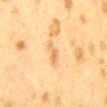Findings:
- workup: imaged on a skin check; not biopsied
- lighting: cross-polarized illumination
- site: the mid back
- patient: female, aged 38–42
- automated lesion analysis: a lesion color around L≈60 a*≈16 b*≈39 in CIELAB and roughly 8 lightness units darker than nearby skin; a border-irregularity rating of about 4/10 and a peripheral color-asymmetry measure near 0.5; a nevus-likeness score of about 0/100 and a lesion-detection confidence of about 100/100
- image source: ~15 mm tile from a whole-body skin photo